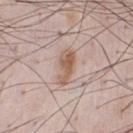Impression:
Recorded during total-body skin imaging; not selected for excision or biopsy.
Background:
Longest diameter approximately 4.5 mm. A region of skin cropped from a whole-body photographic capture, roughly 15 mm wide. A male subject about 35 years old. Imaged with white-light lighting. From the chest.